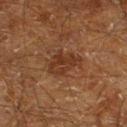biopsy_status: not biopsied; imaged during a skin examination
site: left lower leg
patient:
  sex: male
  age_approx: 60
image:
  source: total-body photography crop
  field_of_view_mm: 15